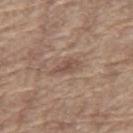| feature | finding |
|---|---|
| follow-up | total-body-photography surveillance lesion; no biopsy |
| TBP lesion metrics | a mean CIELAB color near L≈50 a*≈18 b*≈25, a lesion–skin lightness drop of about 8, and a normalized lesion–skin contrast near 6 |
| patient | male, in their mid- to late 60s |
| location | the mid back |
| lesion diameter | ~3.5 mm (longest diameter) |
| image | ~15 mm crop, total-body skin-cancer survey |
| lighting | white-light illumination |